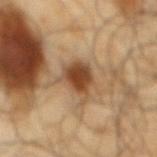Q: Was this lesion biopsied?
A: imaged on a skin check; not biopsied
Q: What kind of image is this?
A: ~15 mm tile from a whole-body skin photo
Q: What did automated image analysis measure?
A: a footprint of about 9 mm² and an outline eccentricity of about 0.6 (0 = round, 1 = elongated); internal color variation of about 4 on a 0–10 scale
Q: Patient demographics?
A: male, aged 63–67
Q: Where on the body is the lesion?
A: the back